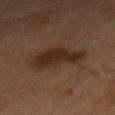Acquisition and patient details: A lesion tile, about 15 mm wide, cut from a 3D total-body photograph. The recorded lesion diameter is about 5 mm. The lesion is on the mid back. This is a cross-polarized tile. A male subject approximately 65 years of age.